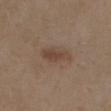Clinical impression: This lesion was catalogued during total-body skin photography and was not selected for biopsy. Clinical summary: This image is a 15 mm lesion crop taken from a total-body photograph. Located on the chest. A female subject, about 40 years old. This is a white-light tile.